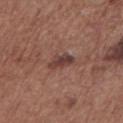This lesion was catalogued during total-body skin photography and was not selected for biopsy.